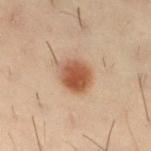Notes:
– biopsy status · no biopsy performed (imaged during a skin exam)
– subject · male, aged around 30
– body site · the right thigh
– acquisition · 15 mm crop, total-body photography
– automated metrics · a lesion area of about 10 mm², a shape eccentricity near 0.4, and a shape-asymmetry score of about 0.15 (0 = symmetric); an average lesion color of about L≈43 a*≈19 b*≈28 (CIELAB) and a lesion–skin lightness drop of about 12
– diameter · ~4 mm (longest diameter)